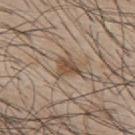A male patient aged around 45. The lesion is on the upper back. A 15 mm close-up extracted from a 3D total-body photography capture.